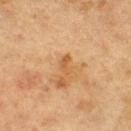The total-body-photography lesion software estimated lesion-presence confidence of about 100/100.
The patient is a female approximately 40 years of age.
Longest diameter approximately 2.5 mm.
Imaged with cross-polarized lighting.
The lesion is on the left thigh.
Cropped from a total-body skin-imaging series; the visible field is about 15 mm.